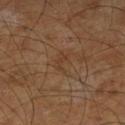<lesion>
<biopsy_status>not biopsied; imaged during a skin examination</biopsy_status>
<site>left lower leg</site>
<lighting>cross-polarized</lighting>
<image>
  <source>total-body photography crop</source>
  <field_of_view_mm>15</field_of_view_mm>
</image>
<patient>
  <sex>male</sex>
  <age_approx>65</age_approx>
</patient>
</lesion>